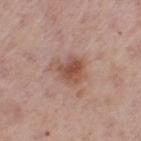Captured during whole-body skin photography for melanoma surveillance; the lesion was not biopsied.
The lesion is on the left thigh.
Cropped from a whole-body photographic skin survey; the tile spans about 15 mm.
Imaged with white-light lighting.
The subject is a female in their mid- to late 60s.
Approximately 4.5 mm at its widest.